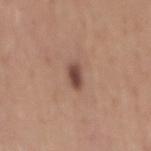Captured during whole-body skin photography for melanoma surveillance; the lesion was not biopsied. The total-body-photography lesion software estimated a footprint of about 4 mm². And it measured roughly 13 lightness units darker than nearby skin. The analysis additionally found a border-irregularity rating of about 2/10, internal color variation of about 3 on a 0–10 scale, and radial color variation of about 1. A lesion tile, about 15 mm wide, cut from a 3D total-body photograph. Longest diameter approximately 2.5 mm. This is a white-light tile. The subject is a male aged around 50. The lesion is on the mid back.